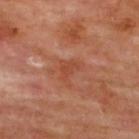Clinical impression: Part of a total-body skin-imaging series; this lesion was reviewed on a skin check and was not flagged for biopsy. Clinical summary: The subject is a male in their mid-60s. The lesion is on the upper back. A 15 mm close-up extracted from a 3D total-body photography capture.